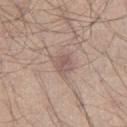{
  "image": {
    "source": "total-body photography crop",
    "field_of_view_mm": 15
  },
  "lesion_size": {
    "long_diameter_mm_approx": 3.0
  },
  "patient": {
    "sex": "male",
    "age_approx": 25
  },
  "site": "right thigh",
  "lighting": "white-light"
}